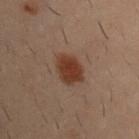The lesion was tiled from a total-body skin photograph and was not biopsied.